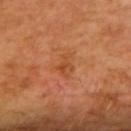Clinical summary:
This is a cross-polarized tile. Cropped from a total-body skin-imaging series; the visible field is about 15 mm. The patient is a female in their mid- to late 40s. Longest diameter approximately 3 mm. The lesion is located on the upper back.